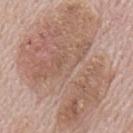Captured during whole-body skin photography for melanoma surveillance; the lesion was not biopsied.
An algorithmic analysis of the crop reported an average lesion color of about L≈59 a*≈18 b*≈26 (CIELAB), roughly 9 lightness units darker than nearby skin, and a normalized border contrast of about 6.5. It also reported a nevus-likeness score of about 0/100 and a detector confidence of about 100 out of 100 that the crop contains a lesion.
The patient is a male aged 68 to 72.
Imaged with white-light lighting.
A 15 mm close-up extracted from a 3D total-body photography capture.
The lesion is on the back.
The recorded lesion diameter is about 15.5 mm.